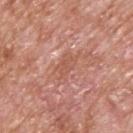No biopsy was performed on this lesion — it was imaged during a full skin examination and was not determined to be concerning.
The subject is a male aged 63 to 67.
Located on the upper back.
A roughly 15 mm field-of-view crop from a total-body skin photograph.
Captured under white-light illumination.
About 3.5 mm across.